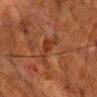{
  "biopsy_status": "not biopsied; imaged during a skin examination",
  "site": "chest",
  "image": {
    "source": "total-body photography crop",
    "field_of_view_mm": 15
  },
  "patient": {
    "sex": "male",
    "age_approx": 65
  },
  "lesion_size": {
    "long_diameter_mm_approx": 4.0
  },
  "lighting": "cross-polarized"
}